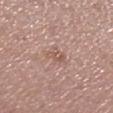The lesion was tiled from a total-body skin photograph and was not biopsied. The subject is a female roughly 60 years of age. From the left lower leg. Cropped from a total-body skin-imaging series; the visible field is about 15 mm. The recorded lesion diameter is about 2.5 mm. Imaged with white-light lighting. Automated tile analysis of the lesion measured a footprint of about 3.5 mm², an eccentricity of roughly 0.7, and two-axis asymmetry of about 0.25. And it measured a lesion color around L≈56 a*≈20 b*≈26 in CIELAB, roughly 7 lightness units darker than nearby skin, and a lesion-to-skin contrast of about 5.5 (normalized; higher = more distinct). The analysis additionally found a classifier nevus-likeness of about 0/100 and a lesion-detection confidence of about 100/100.